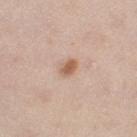| key | value |
|---|---|
| subject | female, aged 38 to 42 |
| anatomic site | the left thigh |
| imaging modality | total-body-photography crop, ~15 mm field of view |